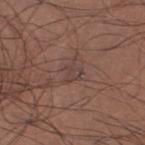Clinical impression:
The lesion was photographed on a routine skin check and not biopsied; there is no pathology result.
Clinical summary:
Measured at roughly 3 mm in maximum diameter. From the leg. The subject is a male in their mid- to late 40s. A 15 mm close-up extracted from a 3D total-body photography capture. The lesion-visualizer software estimated a border-irregularity rating of about 6/10, a within-lesion color-variation index near 1.5/10, and radial color variation of about 0.5. Imaged with white-light lighting.